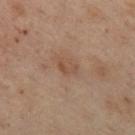The lesion was tiled from a total-body skin photograph and was not biopsied.
From the chest.
A female patient, in their mid- to late 50s.
About 2.5 mm across.
A 15 mm crop from a total-body photograph taken for skin-cancer surveillance.
This is a cross-polarized tile.
An algorithmic analysis of the crop reported a footprint of about 3 mm² and two-axis asymmetry of about 0.25.